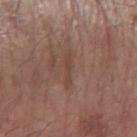Context:
The subject is a male aged 78 to 82. A 15 mm close-up tile from a total-body photography series done for melanoma screening. The recorded lesion diameter is about 3.5 mm. Imaged with white-light lighting. Automated tile analysis of the lesion measured a shape eccentricity near 0.95. And it measured roughly 6 lightness units darker than nearby skin and a lesion-to-skin contrast of about 5 (normalized; higher = more distinct). And it measured a border-irregularity rating of about 5.5/10, a color-variation rating of about 0/10, and a peripheral color-asymmetry measure near 0. The software also gave a lesion-detection confidence of about 100/100. Located on the right forearm.